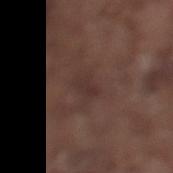Assessment: Captured during whole-body skin photography for melanoma surveillance; the lesion was not biopsied. Acquisition and patient details: A male subject in their mid-70s. The tile uses white-light illumination. A 15 mm crop from a total-body photograph taken for skin-cancer surveillance. On the left lower leg. Approximately 3 mm at its widest.